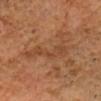Image and clinical context: Located on the head or neck. This is a cross-polarized tile. A roughly 15 mm field-of-view crop from a total-body skin photograph. A male subject, about 60 years old. Automated tile analysis of the lesion measured an automated nevus-likeness rating near 0 out of 100 and a detector confidence of about 85 out of 100 that the crop contains a lesion.